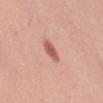• follow-up: imaged on a skin check; not biopsied
• patient: female, in their mid-30s
• body site: the left upper arm
• image source: ~15 mm crop, total-body skin-cancer survey